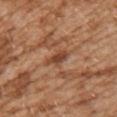Q: Is there a histopathology result?
A: total-body-photography surveillance lesion; no biopsy
Q: Patient demographics?
A: female, aged 28 to 32
Q: How was this image acquired?
A: total-body-photography crop, ~15 mm field of view
Q: Where on the body is the lesion?
A: the chest